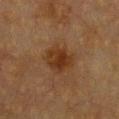No biopsy was performed on this lesion — it was imaged during a full skin examination and was not determined to be concerning. This image is a 15 mm lesion crop taken from a total-body photograph. A female patient in their mid- to late 50s. The lesion is located on the chest. The lesion's longest dimension is about 4 mm. The total-body-photography lesion software estimated an outline eccentricity of about 0.35 (0 = round, 1 = elongated) and two-axis asymmetry of about 0.2. And it measured a border-irregularity rating of about 2/10, a within-lesion color-variation index near 3.5/10, and radial color variation of about 1. The software also gave a nevus-likeness score of about 85/100 and a lesion-detection confidence of about 100/100.